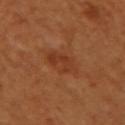Part of a total-body skin-imaging series; this lesion was reviewed on a skin check and was not flagged for biopsy. On the right upper arm. Cropped from a whole-body photographic skin survey; the tile spans about 15 mm. A female patient in their mid- to late 50s. Approximately 3.5 mm at its widest. The tile uses cross-polarized illumination.